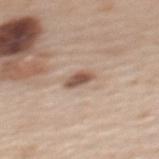Q: Was a biopsy performed?
A: catalogued during a skin exam; not biopsied
Q: What are the patient's age and sex?
A: female, aged approximately 50
Q: Lesion size?
A: ~3 mm (longest diameter)
Q: What kind of image is this?
A: 15 mm crop, total-body photography
Q: Lesion location?
A: the upper back
Q: What did automated image analysis measure?
A: a footprint of about 4 mm², an eccentricity of roughly 0.85, and a symmetry-axis asymmetry near 0.25